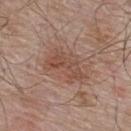The lesion was tiled from a total-body skin photograph and was not biopsied. From the upper back. Captured under white-light illumination. A male patient in their mid-50s. A 15 mm close-up extracted from a 3D total-body photography capture. Approximately 5 mm at its widest.